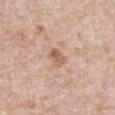workup: no biopsy performed (imaged during a skin exam) | tile lighting: white-light illumination | subject: male, in their 60s | diameter: ≈2.5 mm | site: the abdomen | image-analysis metrics: two-axis asymmetry of about 0.2 | imaging modality: ~15 mm crop, total-body skin-cancer survey.